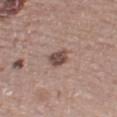Clinical impression: Captured during whole-body skin photography for melanoma surveillance; the lesion was not biopsied. Image and clinical context: Captured under white-light illumination. A 15 mm close-up tile from a total-body photography series done for melanoma screening. A male subject aged around 65. The lesion is located on the left thigh. About 3 mm across. The total-body-photography lesion software estimated a border-irregularity rating of about 1.5/10 and a within-lesion color-variation index near 3/10.